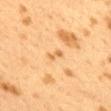Clinical impression: The lesion was tiled from a total-body skin photograph and was not biopsied. Image and clinical context: Located on the mid back. This image is a 15 mm lesion crop taken from a total-body photograph. A female patient roughly 40 years of age. The recorded lesion diameter is about 2.5 mm.